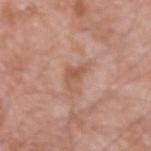• diameter · ≈3.5 mm
• image-analysis metrics · a footprint of about 4.5 mm², a shape eccentricity near 0.8, and two-axis asymmetry of about 0.55; lesion-presence confidence of about 100/100
• acquisition · total-body-photography crop, ~15 mm field of view
• body site · the right forearm
• tile lighting · white-light
• subject · male, aged 58 to 62